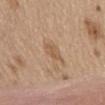Assessment: Part of a total-body skin-imaging series; this lesion was reviewed on a skin check and was not flagged for biopsy. Image and clinical context: The total-body-photography lesion software estimated an average lesion color of about L≈56 a*≈18 b*≈34 (CIELAB) and about 8 CIELAB-L* units darker than the surrounding skin. And it measured a border-irregularity index near 2.5/10. The analysis additionally found a detector confidence of about 100 out of 100 that the crop contains a lesion. Imaged with white-light lighting. The lesion is on the front of the torso. Cropped from a whole-body photographic skin survey; the tile spans about 15 mm. The lesion's longest dimension is about 2.5 mm. A male subject in their 70s.